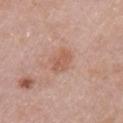Assessment:
The lesion was photographed on a routine skin check and not biopsied; there is no pathology result.